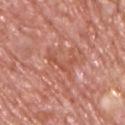Case summary:
• biopsy status · catalogued during a skin exam; not biopsied
• location · the back
• image-analysis metrics · an area of roughly 5.5 mm², an eccentricity of roughly 0.9, and a symmetry-axis asymmetry near 0.6; a lesion color around L≈54 a*≈28 b*≈32 in CIELAB
• diameter · ~5 mm (longest diameter)
• subject · male, about 75 years old
• image source · 15 mm crop, total-body photography